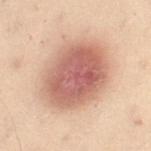follow-up = imaged on a skin check; not biopsied
automated metrics = a lesion area of about 38 mm², an eccentricity of roughly 0.6, and a symmetry-axis asymmetry near 0.1; a within-lesion color-variation index near 6/10 and radial color variation of about 1.5
image source = ~15 mm tile from a whole-body skin photo
subject = female, aged around 50
body site = the right thigh
tile lighting = cross-polarized
lesion size = ≈8 mm